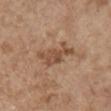| feature | finding |
|---|---|
| workup | total-body-photography surveillance lesion; no biopsy |
| image source | 15 mm crop, total-body photography |
| lighting | white-light |
| patient | female, in their mid- to late 60s |
| diameter | about 5.5 mm |
| site | the right upper arm |